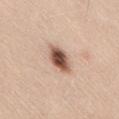workup: imaged on a skin check; not biopsied
location: the lower back
acquisition: total-body-photography crop, ~15 mm field of view
lighting: white-light illumination
patient: male, aged 38 to 42
size: ≈4 mm